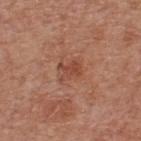Case summary:
– biopsy status · imaged on a skin check; not biopsied
– image · total-body-photography crop, ~15 mm field of view
– anatomic site · the back
– subject · male, in their mid- to late 60s
– size · about 3 mm
– automated lesion analysis · an area of roughly 4.5 mm² and a shape-asymmetry score of about 0.35 (0 = symmetric); a mean CIELAB color near L≈46 a*≈26 b*≈30, roughly 8 lightness units darker than nearby skin, and a lesion-to-skin contrast of about 6 (normalized; higher = more distinct); border irregularity of about 5 on a 0–10 scale, a within-lesion color-variation index near 1.5/10, and peripheral color asymmetry of about 0.5; lesion-presence confidence of about 100/100
– illumination · white-light